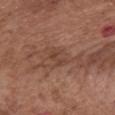Q: Was a biopsy performed?
A: total-body-photography surveillance lesion; no biopsy
Q: Automated lesion metrics?
A: an area of roughly 3.5 mm², an eccentricity of roughly 0.75, and two-axis asymmetry of about 0.45; a lesion–skin lightness drop of about 6 and a lesion-to-skin contrast of about 5.5 (normalized; higher = more distinct)
Q: What is the imaging modality?
A: 15 mm crop, total-body photography
Q: What is the anatomic site?
A: the front of the torso
Q: Illumination type?
A: white-light illumination
Q: What is the lesion's diameter?
A: ≈2.5 mm
Q: Who is the patient?
A: female, in their mid- to late 70s